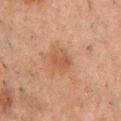Imaged during a routine full-body skin examination; the lesion was not biopsied and no histopathology is available.
Longest diameter approximately 2.5 mm.
The lesion-visualizer software estimated a normalized lesion–skin contrast near 5.5. And it measured an automated nevus-likeness rating near 5 out of 100 and a detector confidence of about 100 out of 100 that the crop contains a lesion.
A male subject aged 48 to 52.
Located on the chest.
A 15 mm close-up extracted from a 3D total-body photography capture.